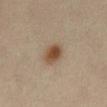notes = no biopsy performed (imaged during a skin exam) | subject = male, about 50 years old | automated lesion analysis = an average lesion color of about L≈48 a*≈16 b*≈30 (CIELAB) and about 12 CIELAB-L* units darker than the surrounding skin; a border-irregularity index near 1.5/10, a within-lesion color-variation index near 4.5/10, and a peripheral color-asymmetry measure near 1.5; a nevus-likeness score of about 100/100 and lesion-presence confidence of about 100/100 | tile lighting = cross-polarized | site = the front of the torso | lesion size = ~3.5 mm (longest diameter) | image = total-body-photography crop, ~15 mm field of view.